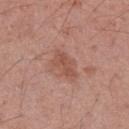  biopsy_status: not biopsied; imaged during a skin examination
  automated_metrics:
    nevus_likeness_0_100: 0
    lesion_detection_confidence_0_100: 100
  lighting: white-light
  patient:
    sex: female
    age_approx: 55
  site: left forearm
  lesion_size:
    long_diameter_mm_approx: 3.5
  image:
    source: total-body photography crop
    field_of_view_mm: 15